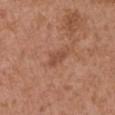biopsy status: imaged on a skin check; not biopsied | subject: male, aged around 75 | acquisition: ~15 mm tile from a whole-body skin photo | lighting: white-light illumination | anatomic site: the left upper arm | lesion size: about 3 mm.